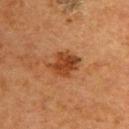This lesion was catalogued during total-body skin photography and was not selected for biopsy. The lesion is located on the upper back. A roughly 15 mm field-of-view crop from a total-body skin photograph. A female patient aged approximately 50. Captured under cross-polarized illumination. The recorded lesion diameter is about 3.5 mm.